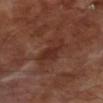<lesion>
<image>
  <source>total-body photography crop</source>
  <field_of_view_mm>15</field_of_view_mm>
</image>
<lesion_size>
  <long_diameter_mm_approx>5.0</long_diameter_mm_approx>
</lesion_size>
<lighting>cross-polarized</lighting>
<automated_metrics>
  <area_mm2_approx>8.5</area_mm2_approx>
  <eccentricity>0.9</eccentricity>
  <shape_asymmetry>0.3</shape_asymmetry>
</automated_metrics>
<site>right lower leg</site>
<patient>
  <sex>male</sex>
  <age_approx>70</age_approx>
</patient>
</lesion>